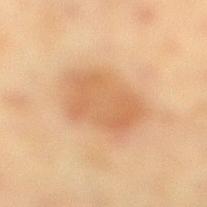Clinical impression:
Captured during whole-body skin photography for melanoma surveillance; the lesion was not biopsied.
Context:
Cropped from a total-body skin-imaging series; the visible field is about 15 mm. The lesion is located on the right lower leg. A female patient, roughly 50 years of age.